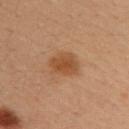Recorded during total-body skin imaging; not selected for excision or biopsy. Located on the upper back. A 15 mm close-up extracted from a 3D total-body photography capture. The subject is a female aged around 35. The lesion's longest dimension is about 3.5 mm.